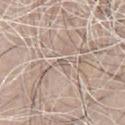{
  "biopsy_status": "not biopsied; imaged during a skin examination",
  "site": "chest",
  "automated_metrics": {
    "border_irregularity_0_10": 2.5,
    "peripheral_color_asymmetry": 0.0
  },
  "image": {
    "source": "total-body photography crop",
    "field_of_view_mm": 15
  },
  "lesion_size": {
    "long_diameter_mm_approx": 1.0
  },
  "patient": {
    "sex": "male",
    "age_approx": 55
  },
  "lighting": "white-light"
}